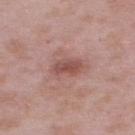workup = total-body-photography surveillance lesion; no biopsy
body site = the back
patient = male, in their mid-50s
acquisition = total-body-photography crop, ~15 mm field of view
tile lighting = white-light illumination